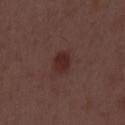notes — imaged on a skin check; not biopsied
image — 15 mm crop, total-body photography
illumination — white-light
subject — male, aged 48–52
size — ~3 mm (longest diameter)